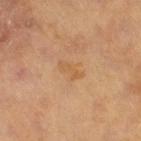notes: imaged on a skin check; not biopsied
subject: male, aged around 65
image: 15 mm crop, total-body photography
illumination: cross-polarized illumination
automated lesion analysis: a border-irregularity index near 4.5/10 and internal color variation of about 0 on a 0–10 scale; an automated nevus-likeness rating near 0 out of 100 and lesion-presence confidence of about 100/100
body site: the left leg
lesion size: ≈3 mm